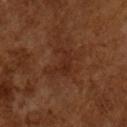The lesion was tiled from a total-body skin photograph and was not biopsied. Automated tile analysis of the lesion measured an average lesion color of about L≈26 a*≈22 b*≈27 (CIELAB) and a normalized lesion–skin contrast near 5.5. It also reported a border-irregularity index near 8/10, internal color variation of about 2 on a 0–10 scale, and a peripheral color-asymmetry measure near 0.5. The analysis additionally found a classifier nevus-likeness of about 0/100 and a detector confidence of about 100 out of 100 that the crop contains a lesion. The subject is a male aged around 65. Imaged with cross-polarized lighting. A 15 mm crop from a total-body photograph taken for skin-cancer surveillance.